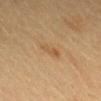Part of a total-body skin-imaging series; this lesion was reviewed on a skin check and was not flagged for biopsy. The lesion is located on the head or neck. The subject is a female in their 20s. An algorithmic analysis of the crop reported an area of roughly 3.5 mm², an eccentricity of roughly 0.9, and a shape-asymmetry score of about 0.25 (0 = symmetric). And it measured a border-irregularity index near 3/10, a within-lesion color-variation index near 1.5/10, and peripheral color asymmetry of about 0.5. The software also gave a nevus-likeness score of about 0/100. About 3 mm across. This is a cross-polarized tile. A lesion tile, about 15 mm wide, cut from a 3D total-body photograph.